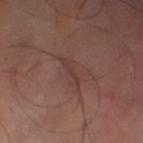• notes: catalogued during a skin exam; not biopsied
• lesion size: about 3.5 mm
• body site: the left thigh
• acquisition: ~15 mm crop, total-body skin-cancer survey
• tile lighting: cross-polarized
• automated metrics: an eccentricity of roughly 0.9 and two-axis asymmetry of about 0.45; lesion-presence confidence of about 55/100